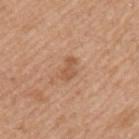Findings:
– notes: catalogued during a skin exam; not biopsied
– subject: male, roughly 55 years of age
– site: the left upper arm
– diameter: about 2.5 mm
– lighting: white-light
– image source: 15 mm crop, total-body photography